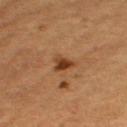Clinical impression: Captured during whole-body skin photography for melanoma surveillance; the lesion was not biopsied. Image and clinical context: Captured under cross-polarized illumination. A female subject, aged 68–72. The lesion is located on the left upper arm. A 15 mm crop from a total-body photograph taken for skin-cancer surveillance.